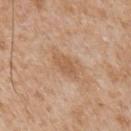{"biopsy_status": "not biopsied; imaged during a skin examination", "lesion_size": {"long_diameter_mm_approx": 4.0}, "patient": {"sex": "male", "age_approx": 65}, "image": {"source": "total-body photography crop", "field_of_view_mm": 15}, "site": "chest", "automated_metrics": {"cielab_L": 58, "cielab_a": 19, "cielab_b": 35, "vs_skin_darker_L": 8.0, "vs_skin_contrast_norm": 6.0, "border_irregularity_0_10": 2.5, "peripheral_color_asymmetry": 0.5, "lesion_detection_confidence_0_100": 100}}